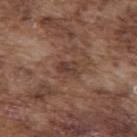This lesion was catalogued during total-body skin photography and was not selected for biopsy.